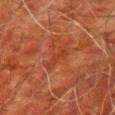Recorded during total-body skin imaging; not selected for excision or biopsy.
The subject is a male in their 80s.
The lesion's longest dimension is about 3 mm.
A close-up tile cropped from a whole-body skin photograph, about 15 mm across.
From the right upper arm.
An algorithmic analysis of the crop reported a mean CIELAB color near L≈32 a*≈26 b*≈30 and a lesion–skin lightness drop of about 5. And it measured a nevus-likeness score of about 0/100 and a detector confidence of about 100 out of 100 that the crop contains a lesion.
This is a cross-polarized tile.